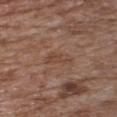Case summary:
• notes · imaged on a skin check; not biopsied
• anatomic site · the chest
• acquisition · 15 mm crop, total-body photography
• subject · female, about 75 years old
• lighting · white-light
• TBP lesion metrics · an eccentricity of roughly 0.9 and a shape-asymmetry score of about 0.35 (0 = symmetric); a nevus-likeness score of about 0/100 and a lesion-detection confidence of about 95/100
• diameter · ≈3.5 mm